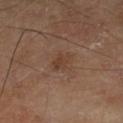Q: Is there a histopathology result?
A: no biopsy performed (imaged during a skin exam)
Q: How was this image acquired?
A: ~15 mm crop, total-body skin-cancer survey
Q: How large is the lesion?
A: ~3 mm (longest diameter)
Q: What is the anatomic site?
A: the left lower leg
Q: Who is the patient?
A: male, aged approximately 65
Q: What lighting was used for the tile?
A: cross-polarized illumination
Q: Automated lesion metrics?
A: an average lesion color of about L≈39 a*≈17 b*≈27 (CIELAB), roughly 6 lightness units darker than nearby skin, and a lesion-to-skin contrast of about 5.5 (normalized; higher = more distinct); an automated nevus-likeness rating near 5 out of 100 and a detector confidence of about 100 out of 100 that the crop contains a lesion